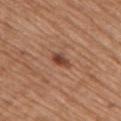Q: Was a biopsy performed?
A: no biopsy performed (imaged during a skin exam)
Q: What is the lesion's diameter?
A: ~3 mm (longest diameter)
Q: Where on the body is the lesion?
A: the upper back
Q: How was this image acquired?
A: total-body-photography crop, ~15 mm field of view
Q: Who is the patient?
A: male, in their 70s
Q: Illumination type?
A: white-light